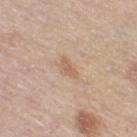Case summary:
• notes — no biopsy performed (imaged during a skin exam)
• anatomic site — the right thigh
• subject — female, aged 63–67
• image source — total-body-photography crop, ~15 mm field of view
• size — ~3 mm (longest diameter)
• tile lighting — white-light illumination